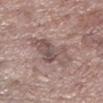notes: imaged on a skin check; not biopsied | anatomic site: the left lower leg | image: ~15 mm crop, total-body skin-cancer survey | patient: female, aged around 85 or older | illumination: white-light illumination | image-analysis metrics: an area of roughly 14 mm² and an outline eccentricity of about 0.75 (0 = round, 1 = elongated); a mean CIELAB color near L≈52 a*≈16 b*≈19, a lesion–skin lightness drop of about 9, and a normalized lesion–skin contrast near 6.5.